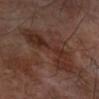Assessment:
Part of a total-body skin-imaging series; this lesion was reviewed on a skin check and was not flagged for biopsy.
Image and clinical context:
A male patient, roughly 70 years of age. The recorded lesion diameter is about 7.5 mm. Automated tile analysis of the lesion measured a lesion area of about 19 mm² and a shape-asymmetry score of about 0.4 (0 = symmetric). It also reported a border-irregularity index near 7.5/10, a color-variation rating of about 4.5/10, and radial color variation of about 1.5. It also reported a classifier nevus-likeness of about 0/100 and lesion-presence confidence of about 100/100. This is a cross-polarized tile. The lesion is on the arm. A 15 mm crop from a total-body photograph taken for skin-cancer surveillance.